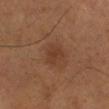Q: Was this lesion biopsied?
A: catalogued during a skin exam; not biopsied
Q: How was this image acquired?
A: 15 mm crop, total-body photography
Q: Where on the body is the lesion?
A: the right lower leg
Q: What lighting was used for the tile?
A: cross-polarized
Q: How large is the lesion?
A: about 3 mm
Q: What are the patient's age and sex?
A: male, approximately 55 years of age